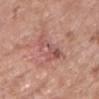Q: Is there a histopathology result?
A: no biopsy performed (imaged during a skin exam)
Q: Automated lesion metrics?
A: an area of roughly 8.5 mm², an outline eccentricity of about 0.8 (0 = round, 1 = elongated), and a symmetry-axis asymmetry near 0.45; a mean CIELAB color near L≈54 a*≈25 b*≈24, roughly 8 lightness units darker than nearby skin, and a normalized border contrast of about 6; border irregularity of about 6 on a 0–10 scale
Q: What are the patient's age and sex?
A: male, approximately 60 years of age
Q: Lesion location?
A: the front of the torso
Q: How was this image acquired?
A: ~15 mm crop, total-body skin-cancer survey